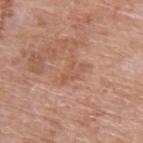biopsy status: catalogued during a skin exam; not biopsied | tile lighting: white-light | imaging modality: 15 mm crop, total-body photography | site: the arm | lesion diameter: ~3.5 mm (longest diameter) | patient: male, roughly 60 years of age.